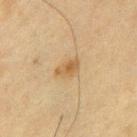Findings:
- follow-up — no biopsy performed (imaged during a skin exam)
- location — the chest
- image source — ~15 mm crop, total-body skin-cancer survey
- subject — male, approximately 75 years of age